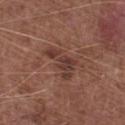This lesion was catalogued during total-body skin photography and was not selected for biopsy.
The tile uses white-light illumination.
A region of skin cropped from a whole-body photographic capture, roughly 15 mm wide.
A male subject, aged 73 to 77.
The recorded lesion diameter is about 4.5 mm.
From the leg.